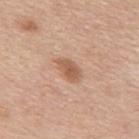<tbp_lesion>
  <biopsy_status>not biopsied; imaged during a skin examination</biopsy_status>
  <image>
    <source>total-body photography crop</source>
    <field_of_view_mm>15</field_of_view_mm>
  </image>
  <lighting>white-light</lighting>
  <patient>
    <sex>male</sex>
    <age_approx>50</age_approx>
  </patient>
  <site>back</site>
  <automated_metrics>
    <border_irregularity_0_10>2.5</border_irregularity_0_10>
    <color_variation_0_10>2.5</color_variation_0_10>
    <nevus_likeness_0_100>70</nevus_likeness_0_100>
  </automated_metrics>
  <lesion_size>
    <long_diameter_mm_approx>3.5</long_diameter_mm_approx>
  </lesion_size>
</tbp_lesion>